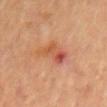{
  "biopsy_status": "not biopsied; imaged during a skin examination"
}